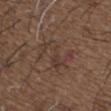Impression:
Imaged during a routine full-body skin examination; the lesion was not biopsied and no histopathology is available.
Background:
A lesion tile, about 15 mm wide, cut from a 3D total-body photograph. From the upper back. A male patient about 50 years old.